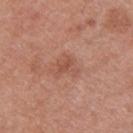Q: Was this lesion biopsied?
A: total-body-photography surveillance lesion; no biopsy
Q: How was this image acquired?
A: 15 mm crop, total-body photography
Q: What is the anatomic site?
A: the upper back
Q: Patient demographics?
A: male, aged 28–32